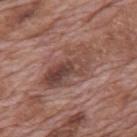Q: Was this lesion biopsied?
A: catalogued during a skin exam; not biopsied
Q: Automated lesion metrics?
A: a border-irregularity rating of about 4/10, a within-lesion color-variation index near 9/10, and radial color variation of about 3.5
Q: How was the tile lit?
A: white-light illumination
Q: What is the lesion's diameter?
A: about 7 mm
Q: What kind of image is this?
A: ~15 mm crop, total-body skin-cancer survey
Q: Where on the body is the lesion?
A: the mid back
Q: What are the patient's age and sex?
A: male, aged around 70